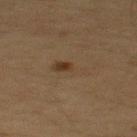No biopsy was performed on this lesion — it was imaged during a full skin examination and was not determined to be concerning. A roughly 15 mm field-of-view crop from a total-body skin photograph. This is a cross-polarized tile. The lesion is located on the upper back. A male patient approximately 65 years of age.